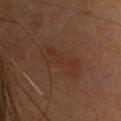This lesion was catalogued during total-body skin photography and was not selected for biopsy.
A female subject aged 28–32.
Imaged with cross-polarized lighting.
On the upper back.
The lesion-visualizer software estimated an area of roughly 10 mm², an eccentricity of roughly 0.95, and a shape-asymmetry score of about 0.3 (0 = symmetric). And it measured an automated nevus-likeness rating near 0 out of 100 and a detector confidence of about 100 out of 100 that the crop contains a lesion.
A 15 mm close-up extracted from a 3D total-body photography capture.
Approximately 5.5 mm at its widest.